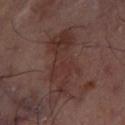Part of a total-body skin-imaging series; this lesion was reviewed on a skin check and was not flagged for biopsy. This image is a 15 mm lesion crop taken from a total-body photograph. Captured under cross-polarized illumination. Longest diameter approximately 9 mm. The lesion is located on the left thigh.